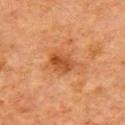Findings:
– notes — imaged on a skin check; not biopsied
– imaging modality — ~15 mm tile from a whole-body skin photo
– lesion diameter — ~3.5 mm (longest diameter)
– subject — male, approximately 80 years of age
– location — the chest